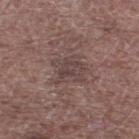Clinical impression: This lesion was catalogued during total-body skin photography and was not selected for biopsy. Background: The lesion is located on the left lower leg. The subject is a male aged 68 to 72. A roughly 15 mm field-of-view crop from a total-body skin photograph. The total-body-photography lesion software estimated a color-variation rating of about 2.5/10 and a peripheral color-asymmetry measure near 1. It also reported a nevus-likeness score of about 0/100 and a detector confidence of about 60 out of 100 that the crop contains a lesion.